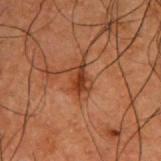Findings:
– biopsy status — imaged on a skin check; not biopsied
– imaging modality — 15 mm crop, total-body photography
– patient — male, roughly 50 years of age
– body site — the upper back
– automated metrics — a footprint of about 3 mm², a shape eccentricity near 0.85, and a shape-asymmetry score of about 0.45 (0 = symmetric); an average lesion color of about L≈28 a*≈22 b*≈28 (CIELAB)
– diameter — about 3 mm
– illumination — cross-polarized